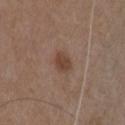{
  "lesion_size": {
    "long_diameter_mm_approx": 2.5
  },
  "patient": {
    "sex": "male",
    "age_approx": 50
  },
  "image": {
    "source": "total-body photography crop",
    "field_of_view_mm": 15
  },
  "automated_metrics": {
    "border_irregularity_0_10": 1.5,
    "color_variation_0_10": 2.5,
    "nevus_likeness_0_100": 90,
    "lesion_detection_confidence_0_100": 100
  },
  "lighting": "white-light",
  "site": "chest"
}